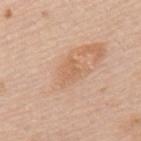Notes:
* acquisition · total-body-photography crop, ~15 mm field of view
* tile lighting · white-light illumination
* body site · the upper back
* automated lesion analysis · an average lesion color of about L≈62 a*≈21 b*≈35 (CIELAB), a lesion–skin lightness drop of about 7, and a normalized border contrast of about 5
* patient · female, aged approximately 65